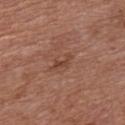Impression: The lesion was tiled from a total-body skin photograph and was not biopsied. Background: A lesion tile, about 15 mm wide, cut from a 3D total-body photograph. The subject is a male in their 70s. The lesion is on the front of the torso. An algorithmic analysis of the crop reported a lesion area of about 2.5 mm², an outline eccentricity of about 0.9 (0 = round, 1 = elongated), and a symmetry-axis asymmetry near 0.5. The software also gave an average lesion color of about L≈43 a*≈23 b*≈28 (CIELAB), roughly 8 lightness units darker than nearby skin, and a normalized border contrast of about 6. And it measured a border-irregularity index near 5.5/10, internal color variation of about 0 on a 0–10 scale, and radial color variation of about 0. And it measured a classifier nevus-likeness of about 0/100 and lesion-presence confidence of about 100/100. The lesion's longest dimension is about 2.5 mm. This is a white-light tile.